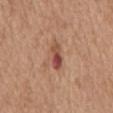This lesion was catalogued during total-body skin photography and was not selected for biopsy.
A female subject, aged approximately 75.
A 15 mm close-up tile from a total-body photography series done for melanoma screening.
The recorded lesion diameter is about 3.5 mm.
On the mid back.
Imaged with white-light lighting.